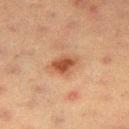Findings:
• lesion diameter — about 3.5 mm
• patient — female, aged 53 to 57
• imaging modality — total-body-photography crop, ~15 mm field of view
• body site — the leg